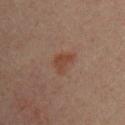{"biopsy_status": "not biopsied; imaged during a skin examination", "lighting": "cross-polarized", "image": {"source": "total-body photography crop", "field_of_view_mm": 15}, "site": "chest", "lesion_size": {"long_diameter_mm_approx": 3.0}, "automated_metrics": {"shape_asymmetry": 0.45, "nevus_likeness_0_100": 20}, "patient": {"sex": "male", "age_approx": 30}}